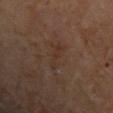Part of a total-body skin-imaging series; this lesion was reviewed on a skin check and was not flagged for biopsy. The subject is a male in their 70s. Located on the arm. A region of skin cropped from a whole-body photographic capture, roughly 15 mm wide. About 4.5 mm across. The tile uses cross-polarized illumination. An algorithmic analysis of the crop reported an eccentricity of roughly 0.95 and two-axis asymmetry of about 0.45. The software also gave a border-irregularity index near 6.5/10. And it measured a nevus-likeness score of about 0/100 and a detector confidence of about 90 out of 100 that the crop contains a lesion.